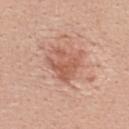Recorded during total-body skin imaging; not selected for excision or biopsy. An algorithmic analysis of the crop reported a footprint of about 11 mm², an eccentricity of roughly 0.3, and two-axis asymmetry of about 0.35. The lesion is on the upper back. A female patient aged approximately 40. The lesion's longest dimension is about 4 mm. This image is a 15 mm lesion crop taken from a total-body photograph. Imaged with white-light lighting.